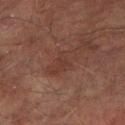<case>
  <biopsy_status>not biopsied; imaged during a skin examination</biopsy_status>
  <patient>
    <sex>male</sex>
    <age_approx>75</age_approx>
  </patient>
  <lighting>cross-polarized</lighting>
  <lesion_size>
    <long_diameter_mm_approx>4.0</long_diameter_mm_approx>
  </lesion_size>
  <site>leg</site>
  <image>
    <source>total-body photography crop</source>
    <field_of_view_mm>15</field_of_view_mm>
  </image>
</case>